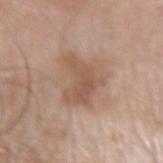The lesion was photographed on a routine skin check and not biopsied; there is no pathology result. About 5 mm across. The subject is a male aged 78 to 82. The lesion-visualizer software estimated a lesion area of about 12 mm², an eccentricity of roughly 0.75, and a shape-asymmetry score of about 0.5 (0 = symmetric). A region of skin cropped from a whole-body photographic capture, roughly 15 mm wide. On the right forearm. Imaged with white-light lighting.